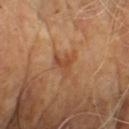The lesion was photographed on a routine skin check and not biopsied; there is no pathology result.
From the chest.
Cropped from a total-body skin-imaging series; the visible field is about 15 mm.
Imaged with cross-polarized lighting.
Automated image analysis of the tile measured an area of roughly 5.5 mm² and a symmetry-axis asymmetry near 0.55. It also reported a nevus-likeness score of about 0/100 and a detector confidence of about 100 out of 100 that the crop contains a lesion.
Measured at roughly 3 mm in maximum diameter.
The patient is a male aged approximately 70.